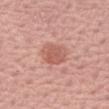Imaged during a routine full-body skin examination; the lesion was not biopsied and no histopathology is available. Cropped from a total-body skin-imaging series; the visible field is about 15 mm. The lesion is located on the right forearm. The patient is a female aged approximately 60. Approximately 3.5 mm at its widest.